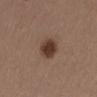Q: Was this lesion biopsied?
A: no biopsy performed (imaged during a skin exam)
Q: Patient demographics?
A: female, in their 40s
Q: How large is the lesion?
A: about 3.5 mm
Q: What lighting was used for the tile?
A: white-light
Q: Lesion location?
A: the abdomen
Q: What is the imaging modality?
A: ~15 mm crop, total-body skin-cancer survey